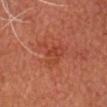Image and clinical context: From the head or neck. Longest diameter approximately 4.5 mm. The tile uses cross-polarized illumination. A 15 mm close-up tile from a total-body photography series done for melanoma screening. A male patient aged 63–67.